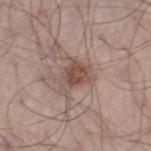patient: male, aged 53 to 57
imaging modality: ~15 mm tile from a whole-body skin photo
body site: the right thigh
lesion size: ≈4.5 mm